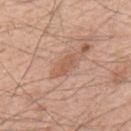Longest diameter approximately 3 mm.
A male subject, aged approximately 55.
The lesion-visualizer software estimated a mean CIELAB color near L≈57 a*≈22 b*≈31. And it measured a border-irregularity rating of about 3.5/10, internal color variation of about 1 on a 0–10 scale, and peripheral color asymmetry of about 0.5. It also reported a classifier nevus-likeness of about 0/100 and a detector confidence of about 95 out of 100 that the crop contains a lesion.
The tile uses white-light illumination.
Cropped from a whole-body photographic skin survey; the tile spans about 15 mm.
On the upper back.